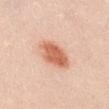biopsy status=catalogued during a skin exam; not biopsied